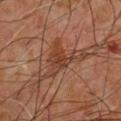The lesion was photographed on a routine skin check and not biopsied; there is no pathology result.